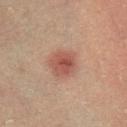Clinical impression: This lesion was catalogued during total-body skin photography and was not selected for biopsy. Context: The lesion is on the right lower leg. A male patient in their mid- to late 70s. This image is a 15 mm lesion crop taken from a total-body photograph.